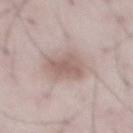notes: total-body-photography surveillance lesion; no biopsy | location: the abdomen | automated lesion analysis: a nevus-likeness score of about 50/100 and lesion-presence confidence of about 90/100 | imaging modality: 15 mm crop, total-body photography | patient: male, aged 48–52.